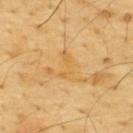Clinical impression: The lesion was tiled from a total-body skin photograph and was not biopsied. Clinical summary: A male patient aged 63–67. The recorded lesion diameter is about 3.5 mm. A lesion tile, about 15 mm wide, cut from a 3D total-body photograph. From the upper back. The tile uses cross-polarized illumination.